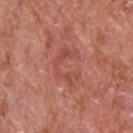The subject is a male in their mid- to late 60s. Longest diameter approximately 4.5 mm. A roughly 15 mm field-of-view crop from a total-body skin photograph. The lesion-visualizer software estimated a mean CIELAB color near L≈50 a*≈29 b*≈28, about 7 CIELAB-L* units darker than the surrounding skin, and a lesion-to-skin contrast of about 5 (normalized; higher = more distinct). It also reported internal color variation of about 2 on a 0–10 scale and radial color variation of about 0.5. The lesion is located on the upper back. The tile uses white-light illumination.